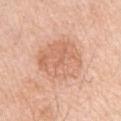• notes · no biopsy performed (imaged during a skin exam)
• site · the arm
• lesion size · ≈5.5 mm
• patient · male, aged around 70
• image source · ~15 mm tile from a whole-body skin photo